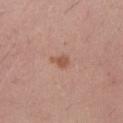The lesion was photographed on a routine skin check and not biopsied; there is no pathology result. A region of skin cropped from a whole-body photographic capture, roughly 15 mm wide. The patient is a female aged around 25. This is a white-light tile. The lesion is on the left forearm.